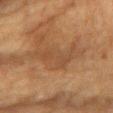Clinical impression: No biopsy was performed on this lesion — it was imaged during a full skin examination and was not determined to be concerning. Acquisition and patient details: A close-up tile cropped from a whole-body skin photograph, about 15 mm across. The total-body-photography lesion software estimated about 6 CIELAB-L* units darker than the surrounding skin and a normalized border contrast of about 5. The analysis additionally found a border-irregularity index near 8/10, a color-variation rating of about 1.5/10, and radial color variation of about 0.5. From the chest. Imaged with cross-polarized lighting. The subject is a male about 85 years old or older. Approximately 6 mm at its widest.